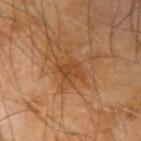{"biopsy_status": "not biopsied; imaged during a skin examination", "image": {"source": "total-body photography crop", "field_of_view_mm": 15}, "lesion_size": {"long_diameter_mm_approx": 3.0}, "site": "right upper arm", "lighting": "cross-polarized", "patient": {"sex": "male", "age_approx": 65}}